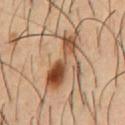follow-up=total-body-photography surveillance lesion; no biopsy
subject=male, roughly 55 years of age
image source=15 mm crop, total-body photography
tile lighting=cross-polarized
TBP lesion metrics=an area of roughly 15 mm², a shape eccentricity near 0.95, and a symmetry-axis asymmetry near 0.35; a nevus-likeness score of about 100/100 and a detector confidence of about 100 out of 100 that the crop contains a lesion
lesion diameter=≈7 mm
anatomic site=the chest